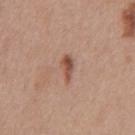notes: total-body-photography surveillance lesion; no biopsy
patient: male, aged 28 to 32
TBP lesion metrics: a border-irregularity index near 4/10, a within-lesion color-variation index near 3/10, and peripheral color asymmetry of about 1; a nevus-likeness score of about 90/100
imaging modality: total-body-photography crop, ~15 mm field of view
body site: the chest